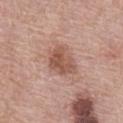notes: imaged on a skin check; not biopsied
imaging modality: total-body-photography crop, ~15 mm field of view
subject: male, aged around 70
diameter: ≈4 mm
site: the chest
image-analysis metrics: an area of roughly 9.5 mm² and a symmetry-axis asymmetry near 0.3; a mean CIELAB color near L≈54 a*≈22 b*≈27, about 11 CIELAB-L* units darker than the surrounding skin, and a normalized border contrast of about 7.5; border irregularity of about 2.5 on a 0–10 scale, a color-variation rating of about 4/10, and radial color variation of about 1.5; an automated nevus-likeness rating near 15 out of 100 and a lesion-detection confidence of about 100/100